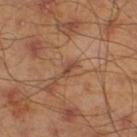| feature | finding |
|---|---|
| follow-up | imaged on a skin check; not biopsied |
| body site | the leg |
| size | ~2.5 mm (longest diameter) |
| lighting | cross-polarized |
| image source | 15 mm crop, total-body photography |
| automated metrics | a footprint of about 2.5 mm² and an eccentricity of roughly 0.85; an average lesion color of about L≈45 a*≈20 b*≈29 (CIELAB), a lesion–skin lightness drop of about 8, and a normalized border contrast of about 6.5 |
| subject | male, aged 43 to 47 |